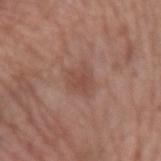Case summary:
* image — ~15 mm crop, total-body skin-cancer survey
* size — ~3.5 mm (longest diameter)
* site — the left forearm
* subject — female, aged around 50
* automated lesion analysis — an area of roughly 7 mm², an eccentricity of roughly 0.5, and two-axis asymmetry of about 0.35; a mean CIELAB color near L≈48 a*≈21 b*≈26 and roughly 7 lightness units darker than nearby skin
* illumination — white-light